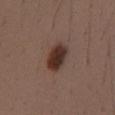Assessment:
Captured during whole-body skin photography for melanoma surveillance; the lesion was not biopsied.
Context:
A male patient, aged 38–42. Longest diameter approximately 4.5 mm. Imaged with white-light lighting. An algorithmic analysis of the crop reported an outline eccentricity of about 0.85 (0 = round, 1 = elongated) and a symmetry-axis asymmetry near 0.15. The software also gave an average lesion color of about L≈33 a*≈17 b*≈23 (CIELAB), roughly 14 lightness units darker than nearby skin, and a lesion-to-skin contrast of about 12 (normalized; higher = more distinct). The software also gave a border-irregularity rating of about 2/10 and a color-variation rating of about 4/10. A region of skin cropped from a whole-body photographic capture, roughly 15 mm wide. Located on the chest.